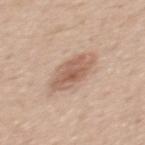Q: Is there a histopathology result?
A: no biopsy performed (imaged during a skin exam)
Q: What is the anatomic site?
A: the mid back
Q: Who is the patient?
A: male, aged approximately 55
Q: Lesion size?
A: about 5.5 mm
Q: What is the imaging modality?
A: 15 mm crop, total-body photography
Q: How was the tile lit?
A: white-light
Q: What did automated image analysis measure?
A: an outline eccentricity of about 0.85 (0 = round, 1 = elongated) and two-axis asymmetry of about 0.15; a mean CIELAB color near L≈59 a*≈19 b*≈28 and a lesion–skin lightness drop of about 11; a lesion-detection confidence of about 100/100